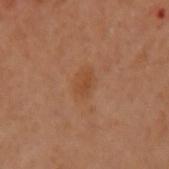Clinical impression:
Captured during whole-body skin photography for melanoma surveillance; the lesion was not biopsied.
Image and clinical context:
On the arm. The patient is a female aged approximately 60. A 15 mm close-up tile from a total-body photography series done for melanoma screening.